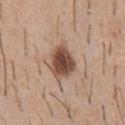notes = imaged on a skin check; not biopsied
acquisition = ~15 mm crop, total-body skin-cancer survey
automated metrics = a lesion color around L≈49 a*≈19 b*≈27 in CIELAB, roughly 16 lightness units darker than nearby skin, and a normalized border contrast of about 11
diameter = about 4 mm
illumination = white-light
location = the chest
patient = male, aged around 40